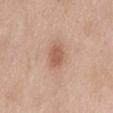follow-up — catalogued during a skin exam; not biopsied | imaging modality — ~15 mm crop, total-body skin-cancer survey | location — the back | tile lighting — white-light illumination | patient — female, approximately 60 years of age.